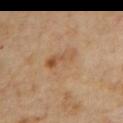• workup: no biopsy performed (imaged during a skin exam)
• location: the back
• acquisition: total-body-photography crop, ~15 mm field of view
• subject: female, in their 60s
• size: ≈4.5 mm
• image-analysis metrics: an outline eccentricity of about 0.75 (0 = round, 1 = elongated) and a symmetry-axis asymmetry near 0.3; an average lesion color of about L≈56 a*≈18 b*≈34 (CIELAB) and a normalized lesion–skin contrast near 4.5
• tile lighting: cross-polarized